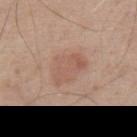Clinical impression: Recorded during total-body skin imaging; not selected for excision or biopsy. Image and clinical context: A male subject, in their 70s. Cropped from a total-body skin-imaging series; the visible field is about 15 mm. Captured under white-light illumination. The total-body-photography lesion software estimated a border-irregularity index near 3/10 and a peripheral color-asymmetry measure near 1. The lesion is on the back. Longest diameter approximately 5 mm.